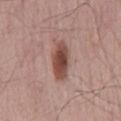Part of a total-body skin-imaging series; this lesion was reviewed on a skin check and was not flagged for biopsy.